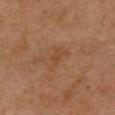No biopsy was performed on this lesion — it was imaged during a full skin examination and was not determined to be concerning. The recorded lesion diameter is about 3 mm. The lesion is located on the front of the torso. This is a cross-polarized tile. A female patient, aged around 60. A 15 mm crop from a total-body photograph taken for skin-cancer surveillance. The lesion-visualizer software estimated an outline eccentricity of about 0.85 (0 = round, 1 = elongated) and two-axis asymmetry of about 0.35. It also reported a border-irregularity rating of about 3.5/10, a color-variation rating of about 1.5/10, and a peripheral color-asymmetry measure near 0.5.